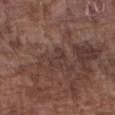| feature | finding |
|---|---|
| biopsy status | total-body-photography surveillance lesion; no biopsy |
| diameter | ~3 mm (longest diameter) |
| patient | male, aged 73–77 |
| body site | the right forearm |
| lighting | white-light |
| acquisition | ~15 mm tile from a whole-body skin photo |